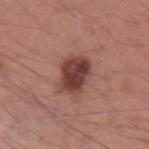Q: Was this lesion biopsied?
A: no biopsy performed (imaged during a skin exam)
Q: What is the imaging modality?
A: 15 mm crop, total-body photography
Q: What lighting was used for the tile?
A: white-light illumination
Q: How large is the lesion?
A: ≈4.5 mm
Q: What is the anatomic site?
A: the left upper arm
Q: Patient demographics?
A: male, roughly 35 years of age